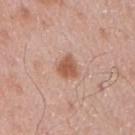Clinical impression:
The lesion was tiled from a total-body skin photograph and was not biopsied.
Image and clinical context:
Cropped from a whole-body photographic skin survey; the tile spans about 15 mm. A male subject approximately 55 years of age. About 3 mm across. From the right upper arm.